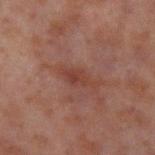A 15 mm close-up tile from a total-body photography series done for melanoma screening.
Captured under cross-polarized illumination.
The lesion is on the left upper arm.
A male patient, in their 30s.
Automated image analysis of the tile measured a symmetry-axis asymmetry near 0.35.
The recorded lesion diameter is about 4.5 mm.